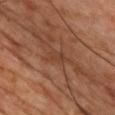The lesion was tiled from a total-body skin photograph and was not biopsied.
The patient is a male aged 53 to 57.
From the chest.
Measured at roughly 3.5 mm in maximum diameter.
A 15 mm close-up tile from a total-body photography series done for melanoma screening.